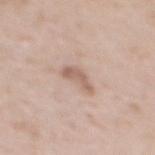{
  "biopsy_status": "not biopsied; imaged during a skin examination",
  "site": "back",
  "image": {
    "source": "total-body photography crop",
    "field_of_view_mm": 15
  },
  "lighting": "white-light",
  "lesion_size": {
    "long_diameter_mm_approx": 3.0
  },
  "automated_metrics": {
    "area_mm2_approx": 4.0,
    "eccentricity": 0.9,
    "shape_asymmetry": 0.55,
    "border_irregularity_0_10": 5.5,
    "color_variation_0_10": 1.0,
    "peripheral_color_asymmetry": 0.5,
    "nevus_likeness_0_100": 5,
    "lesion_detection_confidence_0_100": 100
  },
  "patient": {
    "sex": "female",
    "age_approx": 40
  }
}